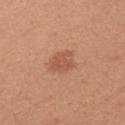The lesion was tiled from a total-body skin photograph and was not biopsied. The lesion is on the left upper arm. A female patient, approximately 20 years of age. The tile uses white-light illumination. A region of skin cropped from a whole-body photographic capture, roughly 15 mm wide. Longest diameter approximately 3.5 mm. Automated image analysis of the tile measured a border-irregularity index near 2.5/10. The software also gave an automated nevus-likeness rating near 45 out of 100 and a lesion-detection confidence of about 100/100.